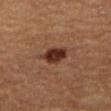image: total-body-photography crop, ~15 mm field of view
automated lesion analysis: a border-irregularity rating of about 2/10, a color-variation rating of about 3/10, and peripheral color asymmetry of about 1; an automated nevus-likeness rating near 100 out of 100
lighting: cross-polarized
anatomic site: the right thigh
lesion diameter: about 3.5 mm
subject: male, aged 58–62